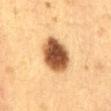notes: total-body-photography surveillance lesion; no biopsy
patient: female, aged approximately 30
body site: the abdomen
lesion size: about 5 mm
image source: ~15 mm tile from a whole-body skin photo